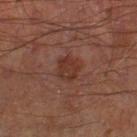| field | value |
|---|---|
| workup | no biopsy performed (imaged during a skin exam) |